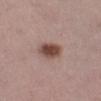Image and clinical context:
A region of skin cropped from a whole-body photographic capture, roughly 15 mm wide. Located on the right lower leg. A female patient, about 25 years old.